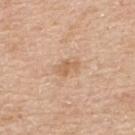{"patient": {"sex": "male", "age_approx": 60}, "image": {"source": "total-body photography crop", "field_of_view_mm": 15}, "site": "upper back", "lighting": "white-light", "lesion_size": {"long_diameter_mm_approx": 2.5}}